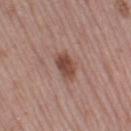{
  "biopsy_status": "not biopsied; imaged during a skin examination",
  "lighting": "white-light",
  "image": {
    "source": "total-body photography crop",
    "field_of_view_mm": 15
  },
  "patient": {
    "sex": "female",
    "age_approx": 40
  },
  "lesion_size": {
    "long_diameter_mm_approx": 3.0
  },
  "site": "left thigh"
}